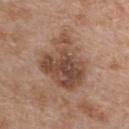On the chest.
A lesion tile, about 15 mm wide, cut from a 3D total-body photograph.
A male patient, aged 63–67.
This is a white-light tile.
About 6.5 mm across.